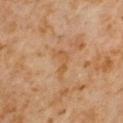Captured during whole-body skin photography for melanoma surveillance; the lesion was not biopsied. A male subject approximately 60 years of age. A 15 mm close-up tile from a total-body photography series done for melanoma screening. The lesion is on the right upper arm. The tile uses cross-polarized illumination. About 3.5 mm across.